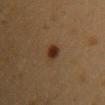| feature | finding |
|---|---|
| follow-up | no biopsy performed (imaged during a skin exam) |
| TBP lesion metrics | a footprint of about 4 mm² and a symmetry-axis asymmetry near 0.2; a border-irregularity rating of about 2/10 and peripheral color asymmetry of about 1.5 |
| subject | female, aged around 30 |
| lighting | cross-polarized illumination |
| image | 15 mm crop, total-body photography |
| location | the chest |